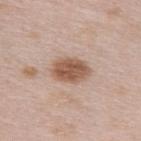On the upper back. The patient is a female approximately 65 years of age. A region of skin cropped from a whole-body photographic capture, roughly 15 mm wide. An algorithmic analysis of the crop reported an area of roughly 10 mm², an eccentricity of roughly 0.75, and two-axis asymmetry of about 0.15. And it measured a mean CIELAB color near L≈56 a*≈19 b*≈28, a lesion–skin lightness drop of about 13, and a lesion-to-skin contrast of about 9 (normalized; higher = more distinct). The software also gave a detector confidence of about 100 out of 100 that the crop contains a lesion. The tile uses white-light illumination.